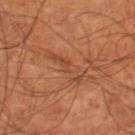Clinical impression: Captured during whole-body skin photography for melanoma surveillance; the lesion was not biopsied. Context: A male subject aged around 60. The lesion is located on the right lower leg. A 15 mm close-up extracted from a 3D total-body photography capture. This is a cross-polarized tile.